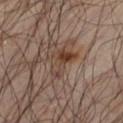Q: Is there a histopathology result?
A: catalogued during a skin exam; not biopsied
Q: What is the anatomic site?
A: the left thigh
Q: What kind of image is this?
A: 15 mm crop, total-body photography
Q: Who is the patient?
A: male, in their mid- to late 60s
Q: What did automated image analysis measure?
A: a lesion area of about 8 mm², an eccentricity of roughly 0.7, and a symmetry-axis asymmetry near 0.5; a mean CIELAB color near L≈39 a*≈16 b*≈24, a lesion–skin lightness drop of about 8, and a normalized lesion–skin contrast near 7.5; lesion-presence confidence of about 100/100